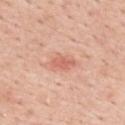Recorded during total-body skin imaging; not selected for excision or biopsy. Longest diameter approximately 3 mm. A female patient aged around 45. This is a white-light tile. Cropped from a total-body skin-imaging series; the visible field is about 15 mm. On the upper back. The total-body-photography lesion software estimated a border-irregularity rating of about 2.5/10, a within-lesion color-variation index near 1.5/10, and radial color variation of about 0.5.